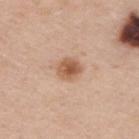  biopsy_status: not biopsied; imaged during a skin examination
  image:
    source: total-body photography crop
    field_of_view_mm: 15
  patient:
    sex: male
    age_approx: 45
  site: upper back